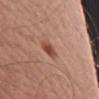Impression: Part of a total-body skin-imaging series; this lesion was reviewed on a skin check and was not flagged for biopsy. Clinical summary: The tile uses white-light illumination. A roughly 15 mm field-of-view crop from a total-body skin photograph. A male subject, approximately 55 years of age. The lesion-visualizer software estimated border irregularity of about 2.5 on a 0–10 scale and a color-variation rating of about 3/10. The software also gave an automated nevus-likeness rating near 85 out of 100. Approximately 2.5 mm at its widest. Located on the right upper arm.